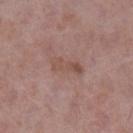Case summary:
– follow-up: no biopsy performed (imaged during a skin exam)
– TBP lesion metrics: a lesion area of about 5.5 mm², an outline eccentricity of about 0.95 (0 = round, 1 = elongated), and two-axis asymmetry of about 0.3; a mean CIELAB color near L≈50 a*≈20 b*≈24 and roughly 7 lightness units darker than nearby skin; a detector confidence of about 100 out of 100 that the crop contains a lesion
– acquisition: total-body-photography crop, ~15 mm field of view
– patient: female, aged 68–72
– diameter: about 4 mm
– illumination: white-light illumination
– location: the left lower leg